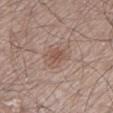Recorded during total-body skin imaging; not selected for excision or biopsy.
An algorithmic analysis of the crop reported a lesion area of about 7.5 mm², an outline eccentricity of about 0.75 (0 = round, 1 = elongated), and a symmetry-axis asymmetry near 0.25. It also reported roughly 7 lightness units darker than nearby skin and a normalized lesion–skin contrast near 5.5.
A close-up tile cropped from a whole-body skin photograph, about 15 mm across.
A male patient about 55 years old.
The lesion is on the left lower leg.